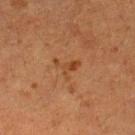Imaged during a routine full-body skin examination; the lesion was not biopsied and no histopathology is available.
This is a cross-polarized tile.
Located on the right lower leg.
Measured at roughly 3 mm in maximum diameter.
A 15 mm close-up tile from a total-body photography series done for melanoma screening.
A female patient aged 48–52.